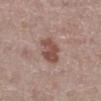Impression: No biopsy was performed on this lesion — it was imaged during a full skin examination and was not determined to be concerning. Acquisition and patient details: A 15 mm crop from a total-body photograph taken for skin-cancer surveillance. The lesion's longest dimension is about 4 mm. The lesion is on the right lower leg. The tile uses white-light illumination. A female patient roughly 50 years of age.